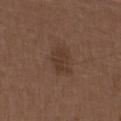| key | value |
|---|---|
| workup | catalogued during a skin exam; not biopsied |